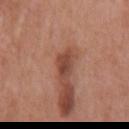biopsy status = catalogued during a skin exam; not biopsied | acquisition = 15 mm crop, total-body photography | patient = male, aged around 70 | lesion size = ≈3 mm | site = the mid back.